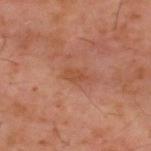This lesion was catalogued during total-body skin photography and was not selected for biopsy.